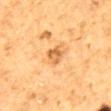Case summary:
- biopsy status · no biopsy performed (imaged during a skin exam)
- automated lesion analysis · an average lesion color of about L≈57 a*≈22 b*≈42 (CIELAB), a lesion–skin lightness drop of about 11, and a normalized border contrast of about 7; a nevus-likeness score of about 5/100
- lesion size · about 3 mm
- image · 15 mm crop, total-body photography
- anatomic site · the mid back
- patient · male, approximately 85 years of age or older
- illumination · cross-polarized illumination